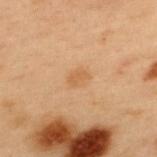Case summary:
• workup: catalogued during a skin exam; not biopsied
• lighting: cross-polarized illumination
• image: ~15 mm crop, total-body skin-cancer survey
• patient: male, in their mid-50s
• size: ≈3 mm
• body site: the upper back